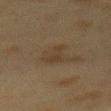Located on the mid back.
A 15 mm close-up tile from a total-body photography series done for melanoma screening.
The tile uses cross-polarized illumination.
A female patient approximately 40 years of age.